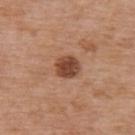automated_metrics:
  area_mm2_approx: 6.0
  eccentricity: 0.4
  shape_asymmetry: 0.1
patient:
  sex: female
  age_approx: 75
lighting: white-light
lesion_size:
  long_diameter_mm_approx: 2.5
site: upper back
image:
  source: total-body photography crop
  field_of_view_mm: 15